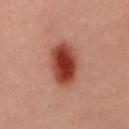Assessment:
Imaged during a routine full-body skin examination; the lesion was not biopsied and no histopathology is available.
Acquisition and patient details:
This is a cross-polarized tile. A male subject, aged 38–42. A lesion tile, about 15 mm wide, cut from a 3D total-body photograph. The recorded lesion diameter is about 6 mm. On the mid back.